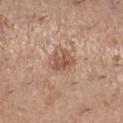| feature | finding |
|---|---|
| notes | imaged on a skin check; not biopsied |
| body site | the right lower leg |
| imaging modality | ~15 mm crop, total-body skin-cancer survey |
| patient | female, aged 28 to 32 |